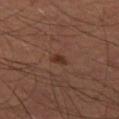Notes:
* tile lighting — cross-polarized
* subject — male, approximately 60 years of age
* body site — the left thigh
* image source — 15 mm crop, total-body photography